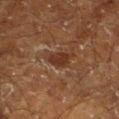Q: Lesion location?
A: the left lower leg
Q: What kind of image is this?
A: ~15 mm crop, total-body skin-cancer survey
Q: How was the tile lit?
A: cross-polarized illumination
Q: Who is the patient?
A: male, about 60 years old
Q: What is the lesion's diameter?
A: about 3 mm